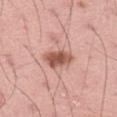Case summary:
* biopsy status · imaged on a skin check; not biopsied
* patient · male, about 45 years old
* image · 15 mm crop, total-body photography
* anatomic site · the lower back
* diameter · ~4 mm (longest diameter)
* tile lighting · white-light